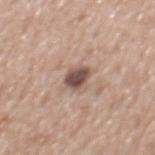* biopsy status — total-body-photography surveillance lesion; no biopsy
* patient — male, in their mid-60s
* imaging modality — ~15 mm crop, total-body skin-cancer survey
* tile lighting — white-light
* anatomic site — the mid back